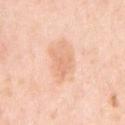  biopsy_status: not biopsied; imaged during a skin examination
  automated_metrics:
    cielab_L: 73
    cielab_a: 23
    cielab_b: 35
    vs_skin_darker_L: 8.0
    vs_skin_contrast_norm: 5.0
  lesion_size:
    long_diameter_mm_approx: 3.5
  site: right upper arm
  image:
    source: total-body photography crop
    field_of_view_mm: 15
  lighting: white-light
  patient:
    sex: female
    age_approx: 65